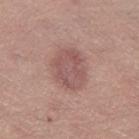Recorded during total-body skin imaging; not selected for excision or biopsy.
About 4.5 mm across.
Located on the left thigh.
This image is a 15 mm lesion crop taken from a total-body photograph.
A female subject approximately 65 years of age.